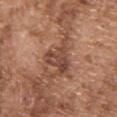<case>
<biopsy_status>not biopsied; imaged during a skin examination</biopsy_status>
<site>upper back</site>
<patient>
  <sex>male</sex>
  <age_approx>75</age_approx>
</patient>
<lesion_size>
  <long_diameter_mm_approx>4.0</long_diameter_mm_approx>
</lesion_size>
<lighting>white-light</lighting>
<image>
  <source>total-body photography crop</source>
  <field_of_view_mm>15</field_of_view_mm>
</image>
</case>